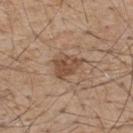No biopsy was performed on this lesion — it was imaged during a full skin examination and was not determined to be concerning.
Longest diameter approximately 4 mm.
Automated image analysis of the tile measured a normalized lesion–skin contrast near 7.5.
The tile uses white-light illumination.
A roughly 15 mm field-of-view crop from a total-body skin photograph.
On the upper back.
A male patient in their mid-50s.